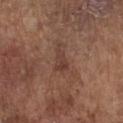Q: Was this lesion biopsied?
A: total-body-photography surveillance lesion; no biopsy
Q: How large is the lesion?
A: ~3 mm (longest diameter)
Q: What lighting was used for the tile?
A: white-light
Q: What are the patient's age and sex?
A: male, in their mid-70s
Q: Lesion location?
A: the front of the torso
Q: What kind of image is this?
A: 15 mm crop, total-body photography